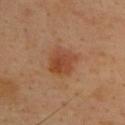{
  "biopsy_status": "not biopsied; imaged during a skin examination",
  "patient": {
    "sex": "male",
    "age_approx": 40
  },
  "image": {
    "source": "total-body photography crop",
    "field_of_view_mm": 15
  },
  "lighting": "cross-polarized",
  "site": "back"
}